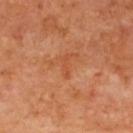Impression:
This lesion was catalogued during total-body skin photography and was not selected for biopsy.
Background:
A female patient aged approximately 40. The lesion is on the back. Cropped from a total-body skin-imaging series; the visible field is about 15 mm.